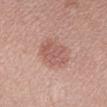| key | value |
|---|---|
| follow-up | imaged on a skin check; not biopsied |
| subject | female, approximately 25 years of age |
| tile lighting | white-light |
| image source | 15 mm crop, total-body photography |
| body site | the right forearm |
| automated metrics | an average lesion color of about L≈57 a*≈23 b*≈25 (CIELAB), about 8 CIELAB-L* units darker than the surrounding skin, and a lesion-to-skin contrast of about 5.5 (normalized; higher = more distinct) |
| lesion size | about 4 mm |